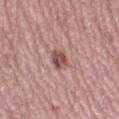Recorded during total-body skin imaging; not selected for excision or biopsy. Approximately 3 mm at its widest. A lesion tile, about 15 mm wide, cut from a 3D total-body photograph. The subject is a female in their 50s. The lesion is on the leg. Automated tile analysis of the lesion measured an eccentricity of roughly 0.8 and two-axis asymmetry of about 0.25. The analysis additionally found a lesion color around L≈50 a*≈22 b*≈23 in CIELAB, a lesion–skin lightness drop of about 13, and a lesion-to-skin contrast of about 9 (normalized; higher = more distinct). Captured under white-light illumination.